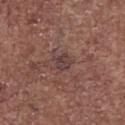Clinical impression: The lesion was photographed on a routine skin check and not biopsied; there is no pathology result. Acquisition and patient details: The total-body-photography lesion software estimated a footprint of about 4.5 mm² and a shape-asymmetry score of about 0.3 (0 = symmetric). It also reported a lesion color around L≈39 a*≈18 b*≈19 in CIELAB and roughly 7 lightness units darker than nearby skin. And it measured an automated nevus-likeness rating near 0 out of 100 and a lesion-detection confidence of about 100/100. Cropped from a total-body skin-imaging series; the visible field is about 15 mm. About 2.5 mm across. This is a white-light tile. The lesion is located on the abdomen. A male subject, approximately 70 years of age.